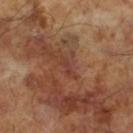Image and clinical context:
Cropped from a total-body skin-imaging series; the visible field is about 15 mm. The recorded lesion diameter is about 2 mm. The patient is a male aged 63 to 67. Imaged with cross-polarized lighting.